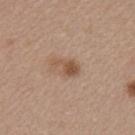Q: Was a biopsy performed?
A: imaged on a skin check; not biopsied
Q: What kind of image is this?
A: ~15 mm crop, total-body skin-cancer survey
Q: Who is the patient?
A: female, aged 43 to 47
Q: Lesion location?
A: the left upper arm
Q: Lesion size?
A: ~3.5 mm (longest diameter)
Q: What lighting was used for the tile?
A: white-light illumination
Q: What did automated image analysis measure?
A: two-axis asymmetry of about 0.35; a mean CIELAB color near L≈53 a*≈18 b*≈30 and a lesion–skin lightness drop of about 10; a border-irregularity index near 4/10 and internal color variation of about 2.5 on a 0–10 scale; a nevus-likeness score of about 55/100 and a lesion-detection confidence of about 100/100